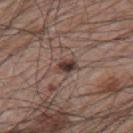| feature | finding |
|---|---|
| follow-up | catalogued during a skin exam; not biopsied |
| patient | male, approximately 65 years of age |
| image | ~15 mm tile from a whole-body skin photo |
| size | ≈2.5 mm |
| site | the left upper arm |